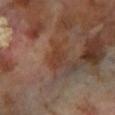The lesion was tiled from a total-body skin photograph and was not biopsied.
A male subject approximately 70 years of age.
Automated image analysis of the tile measured a lesion area of about 4 mm², an outline eccentricity of about 0.7 (0 = round, 1 = elongated), and a symmetry-axis asymmetry near 0.3. The analysis additionally found a classifier nevus-likeness of about 5/100 and a detector confidence of about 100 out of 100 that the crop contains a lesion.
On the left lower leg.
The recorded lesion diameter is about 3 mm.
A 15 mm close-up tile from a total-body photography series done for melanoma screening.